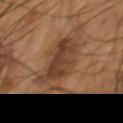Findings:
* notes — imaged on a skin check; not biopsied
* location — the chest
* subject — male, in their mid-50s
* automated lesion analysis — a lesion area of about 28 mm² and a symmetry-axis asymmetry near 0.25; border irregularity of about 6.5 on a 0–10 scale, internal color variation of about 4.5 on a 0–10 scale, and a peripheral color-asymmetry measure near 1.5
* illumination — cross-polarized illumination
* lesion size — about 8 mm
* acquisition — ~15 mm tile from a whole-body skin photo